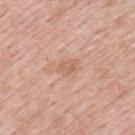Recorded during total-body skin imaging; not selected for excision or biopsy. A lesion tile, about 15 mm wide, cut from a 3D total-body photograph. Located on the upper back. A male subject, aged approximately 55. Approximately 2.5 mm at its widest. Captured under white-light illumination.